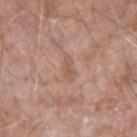biopsy_status: not biopsied; imaged during a skin examination
lighting: white-light
lesion_size:
  long_diameter_mm_approx: 3.0
patient:
  sex: male
  age_approx: 75
image:
  source: total-body photography crop
  field_of_view_mm: 15
site: right forearm
automated_metrics:
  area_mm2_approx: 3.0
  eccentricity: 0.9
  shape_asymmetry: 0.3
  cielab_L: 55
  cielab_a: 20
  cielab_b: 27
  vs_skin_darker_L: 7.0